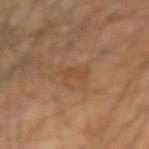Clinical impression: The lesion was tiled from a total-body skin photograph and was not biopsied. Image and clinical context: Longest diameter approximately 3 mm. The lesion is located on the right upper arm. An algorithmic analysis of the crop reported a footprint of about 3.5 mm², an outline eccentricity of about 0.85 (0 = round, 1 = elongated), and a symmetry-axis asymmetry near 0.45. It also reported a border-irregularity index near 6/10, a color-variation rating of about 0/10, and peripheral color asymmetry of about 0. The software also gave a nevus-likeness score of about 0/100 and a lesion-detection confidence of about 100/100. A male patient approximately 65 years of age. Captured under cross-polarized illumination. This image is a 15 mm lesion crop taken from a total-body photograph.